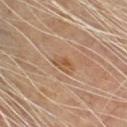This lesion was catalogued during total-body skin photography and was not selected for biopsy.
A 15 mm close-up extracted from a 3D total-body photography capture.
The recorded lesion diameter is about 2.5 mm.
A male subject aged 63–67.
The tile uses cross-polarized illumination.
The lesion is located on the front of the torso.
Automated image analysis of the tile measured a footprint of about 3.5 mm², an eccentricity of roughly 0.8, and a symmetry-axis asymmetry near 0.3. It also reported border irregularity of about 3 on a 0–10 scale, a color-variation rating of about 3/10, and radial color variation of about 1. The software also gave a nevus-likeness score of about 35/100 and lesion-presence confidence of about 100/100.